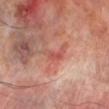Impression: Part of a total-body skin-imaging series; this lesion was reviewed on a skin check and was not flagged for biopsy. Acquisition and patient details: Captured under cross-polarized illumination. Cropped from a total-body skin-imaging series; the visible field is about 15 mm. A male patient aged 68 to 72. The lesion is on the right lower leg.